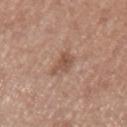workup: total-body-photography surveillance lesion; no biopsy
size: ≈3 mm
location: the left upper arm
subject: female, roughly 50 years of age
image-analysis metrics: a mean CIELAB color near L≈52 a*≈20 b*≈28 and a lesion-to-skin contrast of about 6.5 (normalized; higher = more distinct); a border-irregularity rating of about 4.5/10, internal color variation of about 2 on a 0–10 scale, and a peripheral color-asymmetry measure near 1; an automated nevus-likeness rating near 10 out of 100 and a lesion-detection confidence of about 100/100
image: ~15 mm crop, total-body skin-cancer survey
tile lighting: white-light